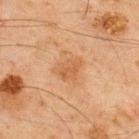No biopsy was performed on this lesion — it was imaged during a full skin examination and was not determined to be concerning.
Automated tile analysis of the lesion measured a footprint of about 5 mm², an outline eccentricity of about 0.65 (0 = round, 1 = elongated), and two-axis asymmetry of about 0.3. And it measured an average lesion color of about L≈47 a*≈20 b*≈34 (CIELAB) and roughly 6 lightness units darker than nearby skin. And it measured border irregularity of about 3 on a 0–10 scale, a within-lesion color-variation index near 2/10, and peripheral color asymmetry of about 0.5.
The lesion is on the upper back.
The lesion's longest dimension is about 3 mm.
Cropped from a total-body skin-imaging series; the visible field is about 15 mm.
A male patient, aged around 45.